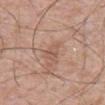Recorded during total-body skin imaging; not selected for excision or biopsy. Automated tile analysis of the lesion measured a footprint of about 3.5 mm² and a shape-asymmetry score of about 0.3 (0 = symmetric). And it measured about 7 CIELAB-L* units darker than the surrounding skin and a normalized lesion–skin contrast near 5. The analysis additionally found an automated nevus-likeness rating near 0 out of 100 and lesion-presence confidence of about 100/100. The patient is a male approximately 70 years of age. A lesion tile, about 15 mm wide, cut from a 3D total-body photograph. Located on the front of the torso.